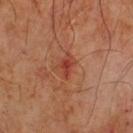follow-up = catalogued during a skin exam; not biopsied
image = ~15 mm tile from a whole-body skin photo
subject = male, aged approximately 65